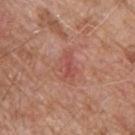{
  "automated_metrics": {
    "area_mm2_approx": 6.5,
    "eccentricity": 0.8,
    "shape_asymmetry": 0.3,
    "border_irregularity_0_10": 3.5,
    "color_variation_0_10": 3.5,
    "peripheral_color_asymmetry": 1.0,
    "nevus_likeness_0_100": 0,
    "lesion_detection_confidence_0_100": 100
  },
  "patient": {
    "sex": "male",
    "age_approx": 80
  },
  "image": {
    "source": "total-body photography crop",
    "field_of_view_mm": 15
  },
  "site": "upper back",
  "lesion_size": {
    "long_diameter_mm_approx": 4.0
  }
}